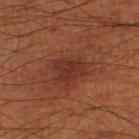Q: What kind of image is this?
A: 15 mm crop, total-body photography
Q: Where on the body is the lesion?
A: the leg
Q: Automated lesion metrics?
A: a footprint of about 8 mm² and an eccentricity of roughly 0.65; a border-irregularity rating of about 3.5/10 and radial color variation of about 1; a nevus-likeness score of about 20/100 and a lesion-detection confidence of about 100/100
Q: How large is the lesion?
A: about 3.5 mm
Q: Patient demographics?
A: male, in their 70s
Q: How was the tile lit?
A: cross-polarized